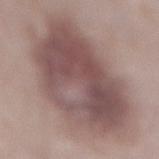{
  "biopsy_status": "not biopsied; imaged during a skin examination",
  "site": "mid back",
  "lesion_size": {
    "long_diameter_mm_approx": 11.5
  },
  "patient": {
    "sex": "male",
    "age_approx": 55
  },
  "lighting": "white-light",
  "image": {
    "source": "total-body photography crop",
    "field_of_view_mm": 15
  }
}